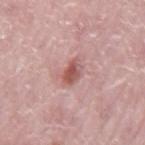On the mid back. An algorithmic analysis of the crop reported a normalized border contrast of about 8.5. The software also gave a border-irregularity rating of about 2/10, internal color variation of about 5.5 on a 0–10 scale, and a peripheral color-asymmetry measure near 2. The tile uses white-light illumination. The recorded lesion diameter is about 3 mm. A male patient in their mid- to late 70s. A 15 mm close-up extracted from a 3D total-body photography capture.